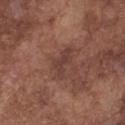notes: catalogued during a skin exam; not biopsied